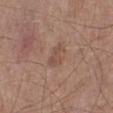Background:
A 15 mm crop from a total-body photograph taken for skin-cancer surveillance. The patient is a male aged approximately 60. The lesion is located on the left lower leg.